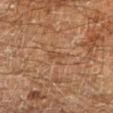<tbp_lesion>
  <lighting>cross-polarized</lighting>
  <image>
    <source>total-body photography crop</source>
    <field_of_view_mm>15</field_of_view_mm>
  </image>
  <patient>
    <sex>male</sex>
    <age_approx>60</age_approx>
  </patient>
  <site>left lower leg</site>
  <lesion_size>
    <long_diameter_mm_approx>2.5</long_diameter_mm_approx>
  </lesion_size>
</tbp_lesion>